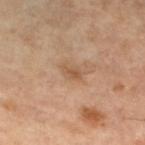| field | value |
|---|---|
| notes | total-body-photography surveillance lesion; no biopsy |
| tile lighting | cross-polarized |
| subject | female, aged approximately 60 |
| imaging modality | total-body-photography crop, ~15 mm field of view |
| anatomic site | the left lower leg |
| size | ~2.5 mm (longest diameter) |